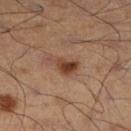follow-up: catalogued during a skin exam; not biopsied | subject: male, about 50 years old | image-analysis metrics: a lesion area of about 5.5 mm², an outline eccentricity of about 0.8 (0 = round, 1 = elongated), and a symmetry-axis asymmetry near 0.45; a mean CIELAB color near L≈41 a*≈20 b*≈29, roughly 11 lightness units darker than nearby skin, and a normalized lesion–skin contrast near 9; a border-irregularity rating of about 4/10 | image source: ~15 mm crop, total-body skin-cancer survey | anatomic site: the left leg | diameter: ≈3.5 mm | illumination: cross-polarized.